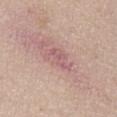Assessment:
Part of a total-body skin-imaging series; this lesion was reviewed on a skin check and was not flagged for biopsy.
Background:
A 15 mm close-up tile from a total-body photography series done for melanoma screening. This is a white-light tile. A female patient aged approximately 60. From the chest.